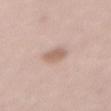Captured during whole-body skin photography for melanoma surveillance; the lesion was not biopsied.
An algorithmic analysis of the crop reported border irregularity of about 2 on a 0–10 scale. It also reported lesion-presence confidence of about 100/100.
The lesion's longest dimension is about 3 mm.
Located on the front of the torso.
The patient is a female aged around 40.
A 15 mm crop from a total-body photograph taken for skin-cancer surveillance.
Captured under white-light illumination.